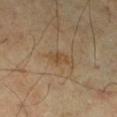A close-up tile cropped from a whole-body skin photograph, about 15 mm across. A male patient aged approximately 70. On the left lower leg. An algorithmic analysis of the crop reported a footprint of about 3.5 mm², a shape eccentricity near 0.85, and a symmetry-axis asymmetry near 0.2. The software also gave a mean CIELAB color near L≈44 a*≈15 b*≈31, a lesion–skin lightness drop of about 6, and a normalized border contrast of about 6. It also reported a nevus-likeness score of about 30/100 and a lesion-detection confidence of about 100/100. Captured under cross-polarized illumination. Measured at roughly 2.5 mm in maximum diameter.